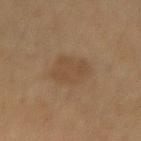The lesion was photographed on a routine skin check and not biopsied; there is no pathology result. Approximately 4 mm at its widest. Automated image analysis of the tile measured an average lesion color of about L≈39 a*≈13 b*≈27 (CIELAB), about 5 CIELAB-L* units darker than the surrounding skin, and a normalized lesion–skin contrast near 5. It also reported a border-irregularity index near 1.5/10, internal color variation of about 2 on a 0–10 scale, and radial color variation of about 0.5. And it measured a classifier nevus-likeness of about 20/100. The subject is a female aged 38 to 42. The lesion is on the left forearm. This is a cross-polarized tile. A close-up tile cropped from a whole-body skin photograph, about 15 mm across.